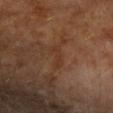<case>
<biopsy_status>not biopsied; imaged during a skin examination</biopsy_status>
<patient>
  <sex>male</sex>
  <age_approx>60</age_approx>
</patient>
<site>left upper arm</site>
<image>
  <source>total-body photography crop</source>
  <field_of_view_mm>15</field_of_view_mm>
</image>
<lighting>cross-polarized</lighting>
<lesion_size>
  <long_diameter_mm_approx>3.5</long_diameter_mm_approx>
</lesion_size>
</case>